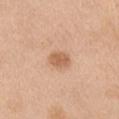Impression: Recorded during total-body skin imaging; not selected for excision or biopsy. Background: Captured under white-light illumination. A region of skin cropped from a whole-body photographic capture, roughly 15 mm wide. A female subject about 55 years old. From the right upper arm. The lesion's longest dimension is about 3 mm.